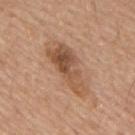Impression:
The lesion was tiled from a total-body skin photograph and was not biopsied.
Clinical summary:
A region of skin cropped from a whole-body photographic capture, roughly 15 mm wide. The lesion is on the upper back. The subject is a male in their mid-60s. This is a white-light tile.